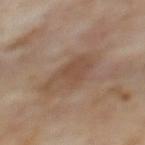notes: imaged on a skin check; not biopsied | image-analysis metrics: a lesion area of about 9 mm² and a shape-asymmetry score of about 0.45 (0 = symmetric); a nevus-likeness score of about 0/100 | diameter: ≈5 mm | image source: ~15 mm tile from a whole-body skin photo | subject: female, in their 60s | body site: the upper back | illumination: cross-polarized.